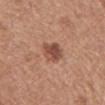No biopsy was performed on this lesion — it was imaged during a full skin examination and was not determined to be concerning. Located on the mid back. The recorded lesion diameter is about 3 mm. A male patient, about 65 years old. Cropped from a total-body skin-imaging series; the visible field is about 15 mm. Captured under white-light illumination.